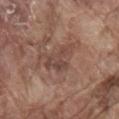Q: Was this lesion biopsied?
A: total-body-photography surveillance lesion; no biopsy
Q: Illumination type?
A: white-light illumination
Q: How large is the lesion?
A: ~5 mm (longest diameter)
Q: Patient demographics?
A: male, aged approximately 80
Q: What is the imaging modality?
A: ~15 mm tile from a whole-body skin photo
Q: Lesion location?
A: the mid back